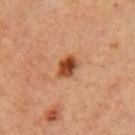Q: Lesion location?
A: the left upper arm
Q: What are the patient's age and sex?
A: female, aged 38 to 42
Q: What is the imaging modality?
A: total-body-photography crop, ~15 mm field of view
Q: What is the lesion's diameter?
A: ~3 mm (longest diameter)
Q: Automated lesion metrics?
A: a border-irregularity index near 2/10, a within-lesion color-variation index near 4.5/10, and radial color variation of about 1.5